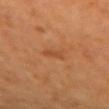Captured during whole-body skin photography for melanoma surveillance; the lesion was not biopsied. The tile uses cross-polarized illumination. A female subject, roughly 40 years of age. From the left forearm. A close-up tile cropped from a whole-body skin photograph, about 15 mm across. Approximately 2.5 mm at its widest.